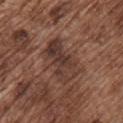The lesion was tiled from a total-body skin photograph and was not biopsied.
The tile uses white-light illumination.
A male patient in their mid- to late 70s.
The lesion-visualizer software estimated a footprint of about 17 mm², an eccentricity of roughly 0.8, and a symmetry-axis asymmetry near 0.25. It also reported a classifier nevus-likeness of about 10/100 and lesion-presence confidence of about 80/100.
The lesion is located on the chest.
The lesion's longest dimension is about 5.5 mm.
A roughly 15 mm field-of-view crop from a total-body skin photograph.